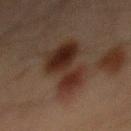Captured during whole-body skin photography for melanoma surveillance; the lesion was not biopsied.
A male subject about 70 years old.
The total-body-photography lesion software estimated border irregularity of about 5.5 on a 0–10 scale, internal color variation of about 6.5 on a 0–10 scale, and peripheral color asymmetry of about 2. It also reported an automated nevus-likeness rating near 25 out of 100 and lesion-presence confidence of about 100/100.
The lesion is located on the abdomen.
This image is a 15 mm lesion crop taken from a total-body photograph.
The recorded lesion diameter is about 8 mm.